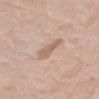biopsy status: imaged on a skin check; not biopsied.